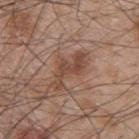Imaged during a routine full-body skin examination; the lesion was not biopsied and no histopathology is available.
A male patient aged 48–52.
About 5 mm across.
A roughly 15 mm field-of-view crop from a total-body skin photograph.
The lesion is on the upper back.
Imaged with white-light lighting.
Automated tile analysis of the lesion measured a footprint of about 8.5 mm², an outline eccentricity of about 0.9 (0 = round, 1 = elongated), and a shape-asymmetry score of about 0.5 (0 = symmetric). And it measured an average lesion color of about L≈46 a*≈20 b*≈27 (CIELAB) and roughly 9 lightness units darker than nearby skin. And it measured border irregularity of about 7 on a 0–10 scale, a color-variation rating of about 2.5/10, and peripheral color asymmetry of about 0.5. The software also gave a nevus-likeness score of about 10/100 and a lesion-detection confidence of about 100/100.